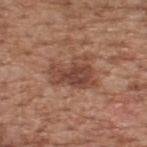workup = catalogued during a skin exam; not biopsied | site = the upper back | diameter = ≈5 mm | illumination = white-light illumination | image source = 15 mm crop, total-body photography | subject = male, aged 63–67.